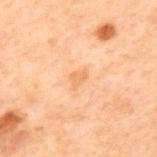<lesion>
<biopsy_status>not biopsied; imaged during a skin examination</biopsy_status>
<automated_metrics>
  <area_mm2_approx>3.0</area_mm2_approx>
  <shape_asymmetry>0.4</shape_asymmetry>
  <cielab_L>55</cielab_L>
  <cielab_a>18</cielab_a>
  <cielab_b>33</cielab_b>
  <vs_skin_darker_L>6.0</vs_skin_darker_L>
  <vs_skin_contrast_norm>4.5</vs_skin_contrast_norm>
</automated_metrics>
<site>upper back</site>
<lesion_size>
  <long_diameter_mm_approx>2.5</long_diameter_mm_approx>
</lesion_size>
<image>
  <source>total-body photography crop</source>
  <field_of_view_mm>15</field_of_view_mm>
</image>
<lighting>cross-polarized</lighting>
<patient>
  <sex>male</sex>
  <age_approx>70</age_approx>
</patient>
</lesion>